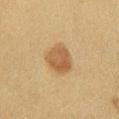follow-up — imaged on a skin check; not biopsied
body site — the left lower leg
imaging modality — total-body-photography crop, ~15 mm field of view
lesion diameter — ~3.5 mm (longest diameter)
patient — female, aged around 55